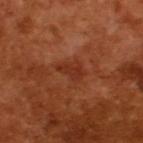notes: catalogued during a skin exam; not biopsied
tile lighting: cross-polarized illumination
subject: male, aged 63 to 67
acquisition: ~15 mm crop, total-body skin-cancer survey